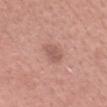follow-up: total-body-photography surveillance lesion; no biopsy
location: the head or neck
automated lesion analysis: a footprint of about 4 mm² and an outline eccentricity of about 0.75 (0 = round, 1 = elongated)
subject: female, in their mid- to late 60s
lesion size: ≈2.5 mm
tile lighting: white-light
imaging modality: ~15 mm crop, total-body skin-cancer survey